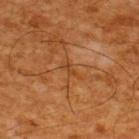{
  "biopsy_status": "not biopsied; imaged during a skin examination",
  "lighting": "cross-polarized",
  "automated_metrics": {
    "cielab_L": 36,
    "cielab_a": 21,
    "cielab_b": 34,
    "vs_skin_darker_L": 5.0,
    "vs_skin_contrast_norm": 5.5,
    "border_irregularity_0_10": 5.0,
    "color_variation_0_10": 0.0
  },
  "lesion_size": {
    "long_diameter_mm_approx": 3.0
  },
  "patient": {
    "sex": "male",
    "age_approx": 65
  },
  "image": {
    "source": "total-body photography crop",
    "field_of_view_mm": 15
  },
  "site": "upper back"
}